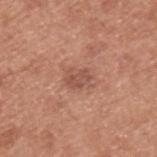Clinical impression:
The lesion was tiled from a total-body skin photograph and was not biopsied.
Context:
A 15 mm crop from a total-body photograph taken for skin-cancer surveillance. On the left upper arm. A male patient, about 55 years old. Imaged with white-light lighting. Longest diameter approximately 2.5 mm.